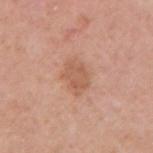Measured at roughly 3.5 mm in maximum diameter.
Captured under white-light illumination.
The subject is a female in their 50s.
A 15 mm close-up extracted from a 3D total-body photography capture.
From the right upper arm.
An algorithmic analysis of the crop reported an area of roughly 8 mm², an outline eccentricity of about 0.7 (0 = round, 1 = elongated), and a shape-asymmetry score of about 0.2 (0 = symmetric). The software also gave an average lesion color of about L≈58 a*≈23 b*≈32 (CIELAB) and roughly 8 lightness units darker than nearby skin. The analysis additionally found a classifier nevus-likeness of about 10/100 and a detector confidence of about 100 out of 100 that the crop contains a lesion.